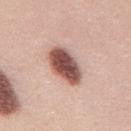Q: Is there a histopathology result?
A: imaged on a skin check; not biopsied
Q: Automated lesion metrics?
A: a classifier nevus-likeness of about 100/100
Q: Lesion location?
A: the mid back
Q: What is the imaging modality?
A: total-body-photography crop, ~15 mm field of view
Q: Illumination type?
A: white-light
Q: Patient demographics?
A: female, approximately 25 years of age
Q: How large is the lesion?
A: about 5.5 mm